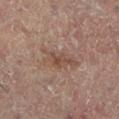Imaged during a routine full-body skin examination; the lesion was not biopsied and no histopathology is available. Automated image analysis of the tile measured a footprint of about 9 mm², an eccentricity of roughly 0.85, and a shape-asymmetry score of about 0.35 (0 = symmetric). The analysis additionally found an average lesion color of about L≈43 a*≈16 b*≈23 (CIELAB), a lesion–skin lightness drop of about 6, and a normalized border contrast of about 5.5. And it measured a border-irregularity rating of about 4.5/10, a color-variation rating of about 4/10, and a peripheral color-asymmetry measure near 1. The subject is a female in their 80s. The lesion is on the leg. A lesion tile, about 15 mm wide, cut from a 3D total-body photograph. This is a cross-polarized tile.